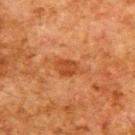{"biopsy_status": "not biopsied; imaged during a skin examination", "automated_metrics": {"area_mm2_approx": 4.0, "eccentricity": 0.65, "shape_asymmetry": 0.2, "vs_skin_darker_L": 8.0, "vs_skin_contrast_norm": 7.0, "nevus_likeness_0_100": 50, "lesion_detection_confidence_0_100": 100}, "patient": {"sex": "male", "age_approx": 80}, "site": "upper back", "lesion_size": {"long_diameter_mm_approx": 2.5}, "image": {"source": "total-body photography crop", "field_of_view_mm": 15}}